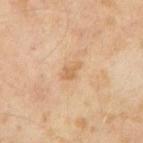Q: Lesion size?
A: ≈2.5 mm
Q: How was this image acquired?
A: total-body-photography crop, ~15 mm field of view
Q: What are the patient's age and sex?
A: male, aged 63 to 67
Q: What lighting was used for the tile?
A: cross-polarized